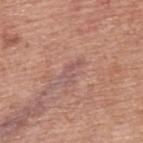| feature | finding |
|---|---|
| workup | imaged on a skin check; not biopsied |
| location | the upper back |
| subject | male, in their mid-50s |
| illumination | white-light |
| acquisition | ~15 mm crop, total-body skin-cancer survey |
| automated metrics | an area of roughly 3 mm², a shape eccentricity near 0.9, and two-axis asymmetry of about 0.65; a normalized lesion–skin contrast near 5; a border-irregularity index near 8/10 and radial color variation of about 0; a detector confidence of about 85 out of 100 that the crop contains a lesion |
| diameter | ≈3 mm |